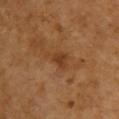Q: Was this lesion biopsied?
A: no biopsy performed (imaged during a skin exam)
Q: Illumination type?
A: cross-polarized
Q: How was this image acquired?
A: ~15 mm tile from a whole-body skin photo
Q: Who is the patient?
A: female, roughly 55 years of age
Q: What is the anatomic site?
A: the upper back
Q: What did automated image analysis measure?
A: a lesion area of about 5 mm², an outline eccentricity of about 0.75 (0 = round, 1 = elongated), and two-axis asymmetry of about 0.45